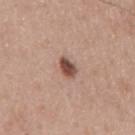biopsy status: no biopsy performed (imaged during a skin exam) | tile lighting: white-light illumination | image source: ~15 mm tile from a whole-body skin photo | body site: the mid back | automated metrics: a lesion area of about 4 mm²; a lesion color around L≈48 a*≈21 b*≈26 in CIELAB and a normalized border contrast of about 11; a color-variation rating of about 3.5/10 and a peripheral color-asymmetry measure near 1; a nevus-likeness score of about 100/100 and a lesion-detection confidence of about 100/100 | subject: male, roughly 60 years of age.